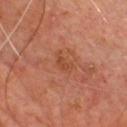  biopsy_status: not biopsied; imaged during a skin examination
  image:
    source: total-body photography crop
    field_of_view_mm: 15
  patient:
    sex: male
    age_approx: 65
  site: front of the torso
  lighting: cross-polarized